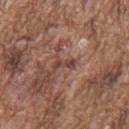Q: Is there a histopathology result?
A: catalogued during a skin exam; not biopsied
Q: Who is the patient?
A: male, approximately 75 years of age
Q: What is the lesion's diameter?
A: ~2.5 mm (longest diameter)
Q: What is the imaging modality?
A: ~15 mm tile from a whole-body skin photo
Q: Where on the body is the lesion?
A: the mid back
Q: Automated lesion metrics?
A: an area of roughly 2.5 mm² and two-axis asymmetry of about 0.35
Q: What lighting was used for the tile?
A: white-light illumination